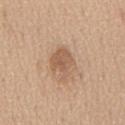The lesion was photographed on a routine skin check and not biopsied; there is no pathology result. The total-body-photography lesion software estimated roughly 10 lightness units darker than nearby skin and a lesion-to-skin contrast of about 6.5 (normalized; higher = more distinct). It also reported a border-irregularity rating of about 3.5/10 and a peripheral color-asymmetry measure near 1. The tile uses white-light illumination. A male patient, aged approximately 60. About 4 mm across. A region of skin cropped from a whole-body photographic capture, roughly 15 mm wide. The lesion is located on the mid back.